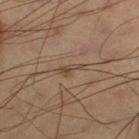Clinical impression: No biopsy was performed on this lesion — it was imaged during a full skin examination and was not determined to be concerning. Background: Cropped from a whole-body photographic skin survey; the tile spans about 15 mm. Imaged with cross-polarized lighting. A male patient aged 58–62. On the left thigh. About 2.5 mm across.